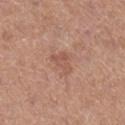workup = imaged on a skin check; not biopsied | patient = female, in their 40s | imaging modality = total-body-photography crop, ~15 mm field of view | location = the right lower leg | lesion size = ≈3 mm.